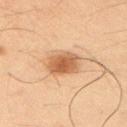A region of skin cropped from a whole-body photographic capture, roughly 15 mm wide. The total-body-photography lesion software estimated an eccentricity of roughly 0.75 and a shape-asymmetry score of about 0.15 (0 = symmetric). And it measured a mean CIELAB color near L≈54 a*≈20 b*≈35 and roughly 12 lightness units darker than nearby skin. The recorded lesion diameter is about 4.5 mm. Captured under cross-polarized illumination. The lesion is on the left upper arm. A male patient, about 35 years old.